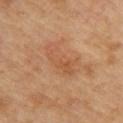Imaged during a routine full-body skin examination; the lesion was not biopsied and no histopathology is available. This is a cross-polarized tile. This image is a 15 mm lesion crop taken from a total-body photograph. The subject is a female aged around 60. Automated image analysis of the tile measured a lesion area of about 9.5 mm², an eccentricity of roughly 0.9, and a shape-asymmetry score of about 0.35 (0 = symmetric). And it measured roughly 6 lightness units darker than nearby skin and a normalized border contrast of about 4.5. The recorded lesion diameter is about 5 mm. The lesion is located on the upper back.